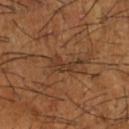biopsy_status: not biopsied; imaged during a skin examination
site: left forearm
image:
  source: total-body photography crop
  field_of_view_mm: 15
patient:
  sex: male
  age_approx: 65